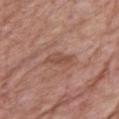Clinical impression:
The lesion was photographed on a routine skin check and not biopsied; there is no pathology result.
Context:
Located on the chest. A 15 mm close-up tile from a total-body photography series done for melanoma screening. The tile uses white-light illumination. A female patient aged 68 to 72. Measured at roughly 3.5 mm in maximum diameter.